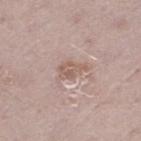Recorded during total-body skin imaging; not selected for excision or biopsy. A male subject, aged approximately 60. On the left thigh. Automated image analysis of the tile measured a mean CIELAB color near L≈58 a*≈17 b*≈25 and a normalized lesion–skin contrast near 6.5. The analysis additionally found a border-irregularity index near 4.5/10, internal color variation of about 3 on a 0–10 scale, and a peripheral color-asymmetry measure near 1.5. A lesion tile, about 15 mm wide, cut from a 3D total-body photograph. This is a white-light tile. About 3 mm across.